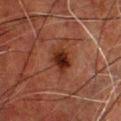| key | value |
|---|---|
| biopsy status | total-body-photography surveillance lesion; no biopsy |
| patient | male, about 50 years old |
| location | the chest |
| image source | 15 mm crop, total-body photography |
| lesion size | about 3 mm |
| image-analysis metrics | an area of roughly 6 mm², an outline eccentricity of about 0.65 (0 = round, 1 = elongated), and a symmetry-axis asymmetry near 0.2; an average lesion color of about L≈23 a*≈21 b*≈24 (CIELAB) and roughly 10 lightness units darker than nearby skin |